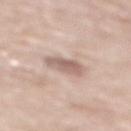Part of a total-body skin-imaging series; this lesion was reviewed on a skin check and was not flagged for biopsy.
The lesion is located on the mid back.
A male patient, approximately 80 years of age.
A 15 mm crop from a total-body photograph taken for skin-cancer surveillance.
Automated image analysis of the tile measured a footprint of about 6.5 mm², a shape eccentricity near 0.8, and two-axis asymmetry of about 0.25. The analysis additionally found a lesion color around L≈61 a*≈17 b*≈24 in CIELAB, roughly 11 lightness units darker than nearby skin, and a normalized border contrast of about 7. And it measured a border-irregularity rating of about 3/10, a color-variation rating of about 2.5/10, and a peripheral color-asymmetry measure near 1. The software also gave a nevus-likeness score of about 0/100 and a lesion-detection confidence of about 100/100.
Measured at roughly 4 mm in maximum diameter.
Imaged with white-light lighting.